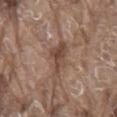Q: Is there a histopathology result?
A: no biopsy performed (imaged during a skin exam)
Q: Lesion location?
A: the mid back
Q: Who is the patient?
A: male, about 80 years old
Q: How was this image acquired?
A: ~15 mm tile from a whole-body skin photo